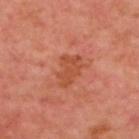The lesion was photographed on a routine skin check and not biopsied; there is no pathology result.
A 15 mm crop from a total-body photograph taken for skin-cancer surveillance.
Measured at roughly 4 mm in maximum diameter.
From the upper back.
A male subject in their 50s.
An algorithmic analysis of the crop reported a lesion area of about 7.5 mm², an outline eccentricity of about 0.75 (0 = round, 1 = elongated), and two-axis asymmetry of about 0.3.